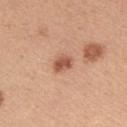notes = total-body-photography surveillance lesion; no biopsy | acquisition = ~15 mm tile from a whole-body skin photo | anatomic site = the left upper arm | lesion size = ~2.5 mm (longest diameter) | lighting = white-light | patient = female, aged 43–47.